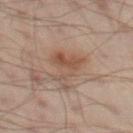Findings:
– image source: 15 mm crop, total-body photography
– TBP lesion metrics: a lesion color around L≈41 a*≈16 b*≈24 in CIELAB, about 7 CIELAB-L* units darker than the surrounding skin, and a normalized border contrast of about 6.5; a color-variation rating of about 4/10 and peripheral color asymmetry of about 1.5
– patient: male, aged 48 to 52
– size: ~4.5 mm (longest diameter)
– site: the left thigh